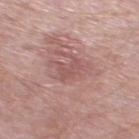Imaged during a routine full-body skin examination; the lesion was not biopsied and no histopathology is available.
A male subject, aged 63–67.
This is a white-light tile.
Automated image analysis of the tile measured a border-irregularity rating of about 4/10 and a color-variation rating of about 1.5/10. The software also gave an automated nevus-likeness rating near 0 out of 100 and a detector confidence of about 95 out of 100 that the crop contains a lesion.
The lesion is on the left thigh.
Cropped from a total-body skin-imaging series; the visible field is about 15 mm.
The recorded lesion diameter is about 3 mm.